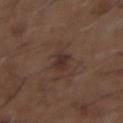Clinical impression: Recorded during total-body skin imaging; not selected for excision or biopsy.